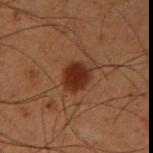A region of skin cropped from a whole-body photographic capture, roughly 15 mm wide.
An algorithmic analysis of the crop reported border irregularity of about 1.5 on a 0–10 scale and a color-variation rating of about 2.5/10. And it measured a nevus-likeness score of about 100/100 and lesion-presence confidence of about 100/100.
A male subject, aged around 55.
Located on the right upper arm.